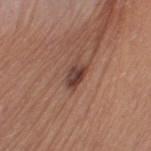Findings:
• tile lighting: white-light
• image: 15 mm crop, total-body photography
• location: the left thigh
• lesion diameter: ≈3 mm
• patient: female, aged approximately 45
• automated metrics: a mean CIELAB color near L≈41 a*≈21 b*≈24, roughly 12 lightness units darker than nearby skin, and a lesion-to-skin contrast of about 10 (normalized; higher = more distinct); a border-irregularity rating of about 3/10, internal color variation of about 4.5 on a 0–10 scale, and peripheral color asymmetry of about 1.5